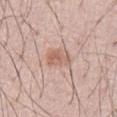No biopsy was performed on this lesion — it was imaged during a full skin examination and was not determined to be concerning.
A male patient, aged 48–52.
A region of skin cropped from a whole-body photographic capture, roughly 15 mm wide.
The lesion is on the front of the torso.